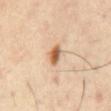Recorded during total-body skin imaging; not selected for excision or biopsy.
A male patient, approximately 40 years of age.
Imaged with cross-polarized lighting.
A roughly 15 mm field-of-view crop from a total-body skin photograph.
From the chest.
The lesion's longest dimension is about 3 mm.